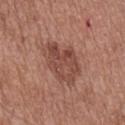Findings:
– workup · imaged on a skin check; not biopsied
– diameter · about 5.5 mm
– acquisition · 15 mm crop, total-body photography
– automated metrics · an eccentricity of roughly 0.8 and a symmetry-axis asymmetry near 0.25; a normalized lesion–skin contrast near 7; border irregularity of about 3 on a 0–10 scale, internal color variation of about 4.5 on a 0–10 scale, and a peripheral color-asymmetry measure near 1.5; an automated nevus-likeness rating near 5 out of 100 and a lesion-detection confidence of about 100/100
– subject · male, about 50 years old
– lighting · white-light
– anatomic site · the mid back